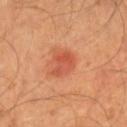The lesion was tiled from a total-body skin photograph and was not biopsied. From the right lower leg. The total-body-photography lesion software estimated a footprint of about 7.5 mm² and a shape-asymmetry score of about 0.3 (0 = symmetric). The analysis additionally found an average lesion color of about L≈55 a*≈33 b*≈38 (CIELAB), a lesion–skin lightness drop of about 9, and a lesion-to-skin contrast of about 6 (normalized; higher = more distinct). It also reported a border-irregularity index near 3/10 and peripheral color asymmetry of about 1.5. Captured under cross-polarized illumination. Measured at roughly 3.5 mm in maximum diameter. A region of skin cropped from a whole-body photographic capture, roughly 15 mm wide. A male patient in their 40s.